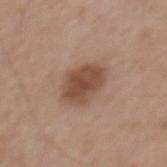workup: no biopsy performed (imaged during a skin exam) | subject: male, approximately 65 years of age | acquisition: ~15 mm crop, total-body skin-cancer survey | location: the mid back.